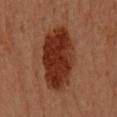No biopsy was performed on this lesion — it was imaged during a full skin examination and was not determined to be concerning. The subject is a female aged approximately 60. Located on the right arm. Imaged with cross-polarized lighting. The recorded lesion diameter is about 7.5 mm. A close-up tile cropped from a whole-body skin photograph, about 15 mm across.